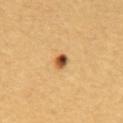Q: Is there a histopathology result?
A: no biopsy performed (imaged during a skin exam)
Q: Lesion size?
A: ≈2 mm
Q: Who is the patient?
A: female, in their 30s
Q: What kind of image is this?
A: ~15 mm crop, total-body skin-cancer survey
Q: What is the anatomic site?
A: the upper back